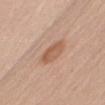The lesion was photographed on a routine skin check and not biopsied; there is no pathology result.
On the right upper arm.
The recorded lesion diameter is about 3 mm.
A 15 mm crop from a total-body photograph taken for skin-cancer surveillance.
Automated image analysis of the tile measured a lesion area of about 6 mm², a shape eccentricity near 0.65, and two-axis asymmetry of about 0.15. It also reported border irregularity of about 1.5 on a 0–10 scale, a within-lesion color-variation index near 3.5/10, and radial color variation of about 1. The software also gave a classifier nevus-likeness of about 70/100 and lesion-presence confidence of about 100/100.
A male subject, approximately 60 years of age.